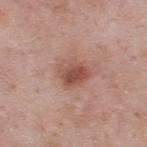Recorded during total-body skin imaging; not selected for excision or biopsy. This is a white-light tile. The lesion's longest dimension is about 3.5 mm. A 15 mm close-up extracted from a 3D total-body photography capture. The patient is a male roughly 55 years of age. Automated image analysis of the tile measured a mean CIELAB color near L≈51 a*≈23 b*≈26, a lesion–skin lightness drop of about 11, and a normalized border contrast of about 7.5. The software also gave radial color variation of about 1.5. The software also gave a nevus-likeness score of about 85/100 and a lesion-detection confidence of about 100/100. From the upper back.